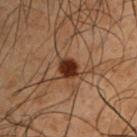Notes:
* biopsy status · imaged on a skin check; not biopsied
* size · ≈2.5 mm
* imaging modality · ~15 mm tile from a whole-body skin photo
* TBP lesion metrics · about 13 CIELAB-L* units darker than the surrounding skin and a normalized border contrast of about 13.5; a border-irregularity rating of about 1/10, a color-variation rating of about 2/10, and radial color variation of about 0.5
* site · the left upper arm
* subject · male, aged 48–52
* illumination · cross-polarized